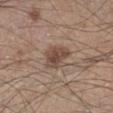Clinical impression:
Part of a total-body skin-imaging series; this lesion was reviewed on a skin check and was not flagged for biopsy.
Image and clinical context:
Captured under white-light illumination. The lesion is located on the leg. Cropped from a whole-body photographic skin survey; the tile spans about 15 mm. A male patient aged approximately 45. Automated tile analysis of the lesion measured a footprint of about 7.5 mm², an outline eccentricity of about 0.7 (0 = round, 1 = elongated), and a symmetry-axis asymmetry near 0.3. The software also gave an average lesion color of about L≈46 a*≈16 b*≈24 (CIELAB), roughly 11 lightness units darker than nearby skin, and a normalized lesion–skin contrast near 8. The software also gave radial color variation of about 1.